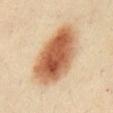<case>
  <biopsy_status>not biopsied; imaged during a skin examination</biopsy_status>
  <image>
    <source>total-body photography crop</source>
    <field_of_view_mm>15</field_of_view_mm>
  </image>
  <lighting>cross-polarized</lighting>
  <lesion_size>
    <long_diameter_mm_approx>9.0</long_diameter_mm_approx>
  </lesion_size>
  <patient>
    <sex>male</sex>
    <age_approx>50</age_approx>
  </patient>
  <site>chest</site>
</case>